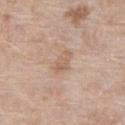workup: catalogued during a skin exam; not biopsied
size: about 3.5 mm
site: the right thigh
image-analysis metrics: a footprint of about 5 mm², an outline eccentricity of about 0.85 (0 = round, 1 = elongated), and two-axis asymmetry of about 0.25; a mean CIELAB color near L≈61 a*≈17 b*≈29 and about 7 CIELAB-L* units darker than the surrounding skin
imaging modality: total-body-photography crop, ~15 mm field of view
lighting: white-light
subject: female, in their mid-70s